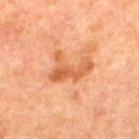| feature | finding |
|---|---|
| notes | imaged on a skin check; not biopsied |
| body site | the upper back |
| size | ~5 mm (longest diameter) |
| patient | male, roughly 60 years of age |
| illumination | cross-polarized |
| imaging modality | ~15 mm tile from a whole-body skin photo |